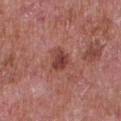No biopsy was performed on this lesion — it was imaged during a full skin examination and was not determined to be concerning. A male subject approximately 65 years of age. A 15 mm close-up extracted from a 3D total-body photography capture. The total-body-photography lesion software estimated a footprint of about 4.5 mm² and an eccentricity of roughly 0.6. The analysis additionally found a lesion–skin lightness drop of about 11 and a lesion-to-skin contrast of about 8.5 (normalized; higher = more distinct). It also reported a border-irregularity index near 2.5/10, a within-lesion color-variation index near 3.5/10, and peripheral color asymmetry of about 1. From the front of the torso. Measured at roughly 2.5 mm in maximum diameter. Captured under white-light illumination.